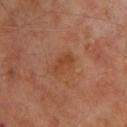Q: Was a biopsy performed?
A: catalogued during a skin exam; not biopsied
Q: How was the tile lit?
A: cross-polarized
Q: What are the patient's age and sex?
A: male, in their mid-60s
Q: Automated lesion metrics?
A: a lesion color around L≈42 a*≈25 b*≈33 in CIELAB, roughly 6 lightness units darker than nearby skin, and a lesion-to-skin contrast of about 6 (normalized; higher = more distinct); a border-irregularity index near 4.5/10, a within-lesion color-variation index near 1.5/10, and a peripheral color-asymmetry measure near 0.5
Q: What is the anatomic site?
A: the upper back
Q: What is the lesion's diameter?
A: ~3 mm (longest diameter)
Q: What kind of image is this?
A: ~15 mm crop, total-body skin-cancer survey